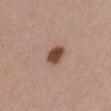Q: Is there a histopathology result?
A: catalogued during a skin exam; not biopsied
Q: Who is the patient?
A: female, aged around 30
Q: How large is the lesion?
A: ~3 mm (longest diameter)
Q: What kind of image is this?
A: ~15 mm tile from a whole-body skin photo
Q: What is the anatomic site?
A: the mid back